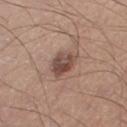<tbp_lesion>
<biopsy_status>not biopsied; imaged during a skin examination</biopsy_status>
<site>right thigh</site>
<image>
  <source>total-body photography crop</source>
  <field_of_view_mm>15</field_of_view_mm>
</image>
<lesion_size>
  <long_diameter_mm_approx>3.5</long_diameter_mm_approx>
</lesion_size>
<lighting>white-light</lighting>
<patient>
  <sex>male</sex>
  <age_approx>40</age_approx>
</patient>
</tbp_lesion>